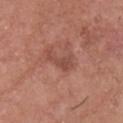Part of a total-body skin-imaging series; this lesion was reviewed on a skin check and was not flagged for biopsy.
Cropped from a total-body skin-imaging series; the visible field is about 15 mm.
On the head or neck.
A male subject, aged 63–67.